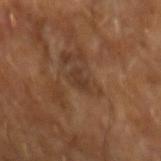Acquisition and patient details: A male patient, about 65 years old. An algorithmic analysis of the crop reported a lesion area of about 3 mm² and a shape eccentricity near 0.85. It also reported a mean CIELAB color near L≈35 a*≈17 b*≈27, roughly 6 lightness units darker than nearby skin, and a normalized lesion–skin contrast near 6. And it measured a border-irregularity rating of about 4/10, a within-lesion color-variation index near 0/10, and a peripheral color-asymmetry measure near 0. And it measured an automated nevus-likeness rating near 0 out of 100. The recorded lesion diameter is about 2.5 mm. This image is a 15 mm lesion crop taken from a total-body photograph.